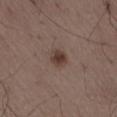<lesion>
<biopsy_status>not biopsied; imaged during a skin examination</biopsy_status>
<lesion_size>
  <long_diameter_mm_approx>3.0</long_diameter_mm_approx>
</lesion_size>
<site>lower back</site>
<image>
  <source>total-body photography crop</source>
  <field_of_view_mm>15</field_of_view_mm>
</image>
<patient>
  <sex>male</sex>
  <age_approx>50</age_approx>
</patient>
</lesion>